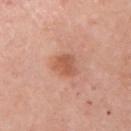patient — female, roughly 65 years of age | automated metrics — an area of roughly 5.5 mm² and two-axis asymmetry of about 0.25; internal color variation of about 2 on a 0–10 scale and peripheral color asymmetry of about 0.5; a classifier nevus-likeness of about 90/100 and a lesion-detection confidence of about 100/100 | location — the right upper arm | illumination — white-light illumination | image source — ~15 mm tile from a whole-body skin photo.